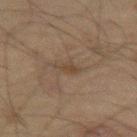{"biopsy_status": "not biopsied; imaged during a skin examination", "image": {"source": "total-body photography crop", "field_of_view_mm": 15}, "lesion_size": {"long_diameter_mm_approx": 3.0}, "automated_metrics": {"area_mm2_approx": 3.5, "shape_asymmetry": 0.3, "nevus_likeness_0_100": 0, "lesion_detection_confidence_0_100": 95}, "lighting": "cross-polarized", "site": "left thigh", "patient": {"sex": "male", "age_approx": 70}}